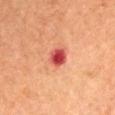  biopsy_status: not biopsied; imaged during a skin examination